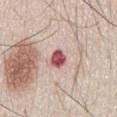{
  "biopsy_status": "not biopsied; imaged during a skin examination",
  "image": {
    "source": "total-body photography crop",
    "field_of_view_mm": 15
  },
  "patient": {
    "sex": "male",
    "age_approx": 45
  },
  "lesion_size": {
    "long_diameter_mm_approx": 2.5
  },
  "site": "chest"
}